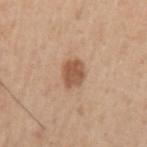Image and clinical context: The lesion's longest dimension is about 3 mm. The lesion is located on the arm. An algorithmic analysis of the crop reported an area of roughly 6.5 mm², an outline eccentricity of about 0.55 (0 = round, 1 = elongated), and a symmetry-axis asymmetry near 0.15. It also reported a mean CIELAB color near L≈54 a*≈21 b*≈32 and about 12 CIELAB-L* units darker than the surrounding skin. This image is a 15 mm lesion crop taken from a total-body photograph. Imaged with white-light lighting. A male patient, aged 53–57.